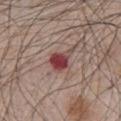The lesion was photographed on a routine skin check and not biopsied; there is no pathology result. The tile uses white-light illumination. A lesion tile, about 15 mm wide, cut from a 3D total-body photograph. About 3.5 mm across. A male subject in their mid- to late 60s. The lesion is located on the chest.